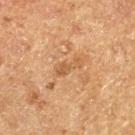notes = no biopsy performed (imaged during a skin exam)
subject = male, aged 73 to 77
body site = the left thigh
image = ~15 mm tile from a whole-body skin photo
illumination = cross-polarized
size = ~3.5 mm (longest diameter)